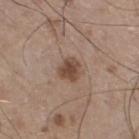This lesion was catalogued during total-body skin photography and was not selected for biopsy.